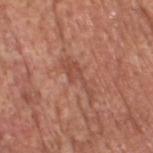biopsy status: catalogued during a skin exam; not biopsied | lesion size: about 5 mm | TBP lesion metrics: a lesion-detection confidence of about 85/100 | acquisition: ~15 mm tile from a whole-body skin photo | subject: male, roughly 65 years of age | illumination: white-light | body site: the head or neck.